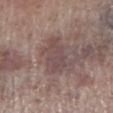notes — catalogued during a skin exam; not biopsied | patient — male, roughly 70 years of age | location — the right lower leg | lesion size — about 5 mm | lighting — white-light illumination | image-analysis metrics — a footprint of about 8.5 mm², an eccentricity of roughly 0.9, and a shape-asymmetry score of about 0.45 (0 = symmetric); a lesion color around L≈46 a*≈17 b*≈17 in CIELAB and a normalized lesion–skin contrast near 6 | acquisition — ~15 mm crop, total-body skin-cancer survey.